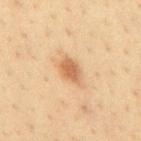Q: Was this lesion biopsied?
A: imaged on a skin check; not biopsied
Q: What lighting was used for the tile?
A: cross-polarized
Q: What kind of image is this?
A: total-body-photography crop, ~15 mm field of view
Q: Lesion location?
A: the mid back
Q: What are the patient's age and sex?
A: male, aged 33–37